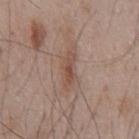The lesion was tiled from a total-body skin photograph and was not biopsied. A 15 mm close-up tile from a total-body photography series done for melanoma screening. A male subject, aged around 55. From the front of the torso.